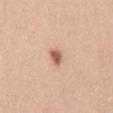A region of skin cropped from a whole-body photographic capture, roughly 15 mm wide. The subject is a female aged approximately 40. From the mid back.